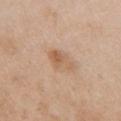Q: Was a biopsy performed?
A: imaged on a skin check; not biopsied
Q: Patient demographics?
A: female, aged 38 to 42
Q: Lesion location?
A: the front of the torso
Q: Automated lesion metrics?
A: a footprint of about 5.5 mm², an eccentricity of roughly 0.85, and a symmetry-axis asymmetry near 0.3; border irregularity of about 3 on a 0–10 scale, internal color variation of about 4.5 on a 0–10 scale, and a peripheral color-asymmetry measure near 1.5
Q: How was the tile lit?
A: white-light illumination
Q: What is the imaging modality?
A: ~15 mm crop, total-body skin-cancer survey
Q: Lesion size?
A: about 4 mm